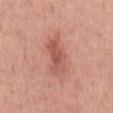No biopsy was performed on this lesion — it was imaged during a full skin examination and was not determined to be concerning. A 15 mm close-up tile from a total-body photography series done for melanoma screening. The lesion-visualizer software estimated a footprint of about 9 mm². The software also gave a border-irregularity index near 4/10 and internal color variation of about 4 on a 0–10 scale. A male patient, aged approximately 50. On the mid back. The recorded lesion diameter is about 5.5 mm.